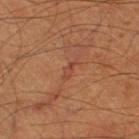Assessment:
Captured during whole-body skin photography for melanoma surveillance; the lesion was not biopsied.
Context:
The tile uses cross-polarized illumination. A male subject aged 58 to 62. Located on the right thigh. A 15 mm crop from a total-body photograph taken for skin-cancer surveillance.